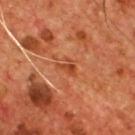Captured during whole-body skin photography for melanoma surveillance; the lesion was not biopsied.
Imaged with cross-polarized lighting.
A male subject about 55 years old.
About 2.5 mm across.
A 15 mm close-up tile from a total-body photography series done for melanoma screening.
On the chest.